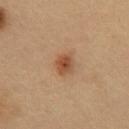Impression:
The lesion was photographed on a routine skin check and not biopsied; there is no pathology result.
Clinical summary:
The tile uses cross-polarized illumination. Measured at roughly 3 mm in maximum diameter. A roughly 15 mm field-of-view crop from a total-body skin photograph. The lesion is located on the abdomen. The subject is a male roughly 50 years of age. An algorithmic analysis of the crop reported an area of roughly 4.5 mm² and a symmetry-axis asymmetry near 0.2. The analysis additionally found an average lesion color of about L≈49 a*≈22 b*≈34 (CIELAB), roughly 11 lightness units darker than nearby skin, and a normalized border contrast of about 8.5. It also reported a within-lesion color-variation index near 4/10 and radial color variation of about 1.5.